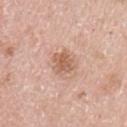  biopsy_status: not biopsied; imaged during a skin examination
  patient:
    sex: female
    age_approx: 50
  site: left upper arm
  image:
    source: total-body photography crop
    field_of_view_mm: 15